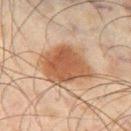Captured during whole-body skin photography for melanoma surveillance; the lesion was not biopsied. On the left thigh. A region of skin cropped from a whole-body photographic capture, roughly 15 mm wide. Imaged with cross-polarized lighting. A male subject, aged 43–47. An algorithmic analysis of the crop reported a lesion-detection confidence of about 100/100. Approximately 7 mm at its widest.